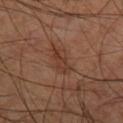biopsy status = no biopsy performed (imaged during a skin exam); diameter = ≈3.5 mm; acquisition = ~15 mm crop, total-body skin-cancer survey; tile lighting = cross-polarized illumination; location = the leg; subject = male, roughly 60 years of age.